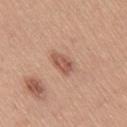Findings:
* follow-up · no biopsy performed (imaged during a skin exam)
* site · the right thigh
* subject · female, aged approximately 55
* TBP lesion metrics · border irregularity of about 2.5 on a 0–10 scale and internal color variation of about 3.5 on a 0–10 scale; a classifier nevus-likeness of about 80/100 and lesion-presence confidence of about 100/100
* imaging modality · 15 mm crop, total-body photography
* lesion size · about 3.5 mm
* tile lighting · white-light